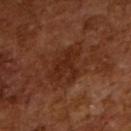biopsy status: no biopsy performed (imaged during a skin exam)
patient: male, in their mid- to late 60s
image source: ~15 mm tile from a whole-body skin photo